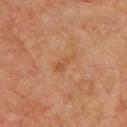<lesion>
<biopsy_status>not biopsied; imaged during a skin examination</biopsy_status>
<lighting>cross-polarized</lighting>
<site>chest</site>
<automated_metrics>
  <cielab_L>51</cielab_L>
  <cielab_a>23</cielab_a>
  <cielab_b>37</cielab_b>
  <vs_skin_contrast_norm>5.5</vs_skin_contrast_norm>
  <nevus_likeness_0_100>0</nevus_likeness_0_100>
  <lesion_detection_confidence_0_100>100</lesion_detection_confidence_0_100>
</automated_metrics>
<image>
  <source>total-body photography crop</source>
  <field_of_view_mm>15</field_of_view_mm>
</image>
<patient>
  <sex>male</sex>
  <age_approx>65</age_approx>
</patient>
<lesion_size>
  <long_diameter_mm_approx>3.0</long_diameter_mm_approx>
</lesion_size>
</lesion>